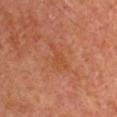Case summary:
- follow-up — catalogued during a skin exam; not biopsied
- image — 15 mm crop, total-body photography
- automated metrics — a footprint of about 4.5 mm², an outline eccentricity of about 0.9 (0 = round, 1 = elongated), and a symmetry-axis asymmetry near 0.55
- body site — the arm
- subject — male, aged around 65
- tile lighting — cross-polarized